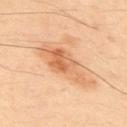biopsy status: imaged on a skin check; not biopsied
imaging modality: ~15 mm crop, total-body skin-cancer survey
lesion size: about 7 mm
site: the upper back
patient: male, aged approximately 50
automated lesion analysis: a border-irregularity rating of about 4/10, a color-variation rating of about 7/10, and radial color variation of about 2.5; a detector confidence of about 100 out of 100 that the crop contains a lesion
lighting: cross-polarized illumination